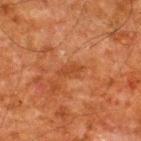Imaged during a routine full-body skin examination; the lesion was not biopsied and no histopathology is available. This is a cross-polarized tile. This image is a 15 mm lesion crop taken from a total-body photograph. Approximately 2.5 mm at its widest. An algorithmic analysis of the crop reported an eccentricity of roughly 0.9 and a shape-asymmetry score of about 0.3 (0 = symmetric). It also reported about 6 CIELAB-L* units darker than the surrounding skin and a normalized border contrast of about 5.5. It also reported border irregularity of about 3.5 on a 0–10 scale, internal color variation of about 0 on a 0–10 scale, and a peripheral color-asymmetry measure near 0. And it measured a classifier nevus-likeness of about 0/100. A male subject, about 60 years old. Located on the upper back.